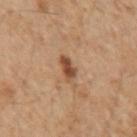This lesion was catalogued during total-body skin photography and was not selected for biopsy. This is a cross-polarized tile. The patient is a female aged around 55. The lesion is on the left forearm. Approximately 2.5 mm at its widest. A region of skin cropped from a whole-body photographic capture, roughly 15 mm wide.